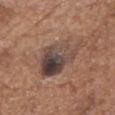| feature | finding |
|---|---|
| workup | catalogued during a skin exam; not biopsied |
| anatomic site | the head or neck |
| patient | male, roughly 80 years of age |
| image-analysis metrics | an eccentricity of roughly 0.7 and a symmetry-axis asymmetry near 0.35; a border-irregularity index near 4/10 |
| lighting | white-light illumination |
| imaging modality | ~15 mm tile from a whole-body skin photo |
| lesion size | about 6.5 mm |